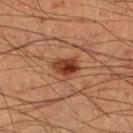Part of a total-body skin-imaging series; this lesion was reviewed on a skin check and was not flagged for biopsy. Approximately 3 mm at its widest. This is a cross-polarized tile. A 15 mm close-up tile from a total-body photography series done for melanoma screening. The patient is a male aged 58 to 62. An algorithmic analysis of the crop reported a lesion area of about 5 mm², an eccentricity of roughly 0.75, and a symmetry-axis asymmetry near 0.3. Located on the right lower leg.